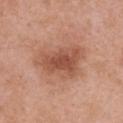| feature | finding |
|---|---|
| follow-up | catalogued during a skin exam; not biopsied |
| acquisition | 15 mm crop, total-body photography |
| anatomic site | the front of the torso |
| TBP lesion metrics | a border-irregularity index near 4/10 and a color-variation rating of about 4/10 |
| subject | female, roughly 60 years of age |